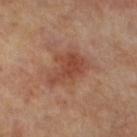Imaged during a routine full-body skin examination; the lesion was not biopsied and no histopathology is available.
The lesion is on the right leg.
A 15 mm close-up extracted from a 3D total-body photography capture.
A female subject, aged 63 to 67.
About 5 mm across.
The tile uses cross-polarized illumination.